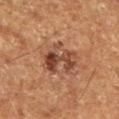{"image": {"source": "total-body photography crop", "field_of_view_mm": 15}, "site": "leg", "patient": {"sex": "male", "age_approx": 65}}